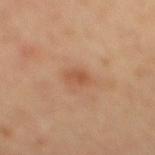• notes · no biopsy performed (imaged during a skin exam)
• tile lighting · cross-polarized
• lesion size · ~2 mm (longest diameter)
• patient · female, roughly 50 years of age
• imaging modality · total-body-photography crop, ~15 mm field of view
• image-analysis metrics · roughly 8 lightness units darker than nearby skin and a lesion-to-skin contrast of about 6 (normalized; higher = more distinct); a lesion-detection confidence of about 100/100
• body site · the mid back